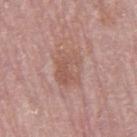follow-up = total-body-photography surveillance lesion; no biopsy
anatomic site = the right thigh
illumination = white-light
patient = female, aged around 65
lesion size = about 4 mm
acquisition = ~15 mm tile from a whole-body skin photo